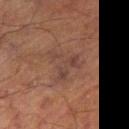This lesion was catalogued during total-body skin photography and was not selected for biopsy.
A close-up tile cropped from a whole-body skin photograph, about 15 mm across.
Measured at roughly 4 mm in maximum diameter.
The lesion-visualizer software estimated a lesion area of about 8 mm² and two-axis asymmetry of about 0.75. The software also gave border irregularity of about 9.5 on a 0–10 scale, a color-variation rating of about 3/10, and peripheral color asymmetry of about 1. And it measured an automated nevus-likeness rating near 0 out of 100 and a lesion-detection confidence of about 100/100.
The lesion is located on the leg.
Imaged with cross-polarized lighting.
The patient is a male approximately 65 years of age.